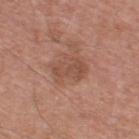| key | value |
|---|---|
| notes | no biopsy performed (imaged during a skin exam) |
| acquisition | ~15 mm tile from a whole-body skin photo |
| size | ~4 mm (longest diameter) |
| patient | male, aged 53 to 57 |
| tile lighting | white-light illumination |
| location | the back |
| automated metrics | a footprint of about 8.5 mm², a shape eccentricity near 0.75, and a shape-asymmetry score of about 0.3 (0 = symmetric) |